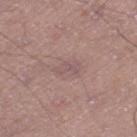Assessment:
This lesion was catalogued during total-body skin photography and was not selected for biopsy.
Acquisition and patient details:
Cropped from a whole-body photographic skin survey; the tile spans about 15 mm. Imaged with white-light lighting. The lesion's longest dimension is about 3 mm. A male patient approximately 55 years of age. An algorithmic analysis of the crop reported a classifier nevus-likeness of about 0/100 and a lesion-detection confidence of about 100/100. On the leg.